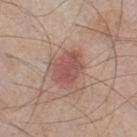Q: Was a biopsy performed?
A: total-body-photography surveillance lesion; no biopsy
Q: Lesion size?
A: about 4 mm
Q: How was this image acquired?
A: ~15 mm tile from a whole-body skin photo
Q: Patient demographics?
A: male, in their 70s
Q: Automated lesion metrics?
A: a footprint of about 9.5 mm², a shape eccentricity near 0.7, and a symmetry-axis asymmetry near 0.2
Q: Lesion location?
A: the right thigh
Q: Illumination type?
A: white-light